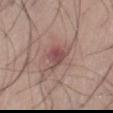follow-up — no biopsy performed (imaged during a skin exam)
image — total-body-photography crop, ~15 mm field of view
body site — the right thigh
lesion size — ≈3.5 mm
patient — male, in their 30s